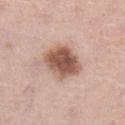Findings:
- workup — imaged on a skin check; not biopsied
- illumination — white-light
- site — the left lower leg
- imaging modality — ~15 mm tile from a whole-body skin photo
- patient — female, in their mid- to late 60s